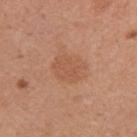Captured during whole-body skin photography for melanoma surveillance; the lesion was not biopsied. A female subject, aged around 35. From the right upper arm. A 15 mm close-up tile from a total-body photography series done for melanoma screening. Measured at roughly 4 mm in maximum diameter. This is a white-light tile.